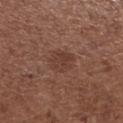follow-up = total-body-photography surveillance lesion; no biopsy.